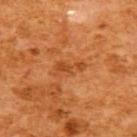The lesion was photographed on a routine skin check and not biopsied; there is no pathology result.
Located on the upper back.
The subject is a female in their mid- to late 50s.
This image is a 15 mm lesion crop taken from a total-body photograph.
Automated image analysis of the tile measured an area of roughly 4.5 mm², an outline eccentricity of about 0.9 (0 = round, 1 = elongated), and a symmetry-axis asymmetry near 0.5. And it measured a border-irregularity rating of about 6.5/10, a within-lesion color-variation index near 1/10, and peripheral color asymmetry of about 0. The software also gave an automated nevus-likeness rating near 0 out of 100 and a detector confidence of about 100 out of 100 that the crop contains a lesion.
This is a cross-polarized tile.